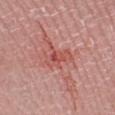Assessment: Part of a total-body skin-imaging series; this lesion was reviewed on a skin check and was not flagged for biopsy. Clinical summary: A female patient, in their mid-50s. Approximately 4 mm at its widest. This image is a 15 mm lesion crop taken from a total-body photograph. This is a white-light tile. Automated tile analysis of the lesion measured a lesion area of about 6 mm² and an eccentricity of roughly 0.8. The software also gave border irregularity of about 7 on a 0–10 scale, a within-lesion color-variation index near 6/10, and peripheral color asymmetry of about 1.5. Located on the left lower leg.